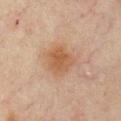Q: Was a biopsy performed?
A: imaged on a skin check; not biopsied
Q: What lighting was used for the tile?
A: cross-polarized
Q: Where on the body is the lesion?
A: the chest
Q: What are the patient's age and sex?
A: male, about 45 years old
Q: How was this image acquired?
A: ~15 mm tile from a whole-body skin photo
Q: What did automated image analysis measure?
A: a classifier nevus-likeness of about 80/100 and a detector confidence of about 100 out of 100 that the crop contains a lesion
Q: Lesion size?
A: ~3.5 mm (longest diameter)